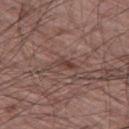workup = catalogued during a skin exam; not biopsied | acquisition = ~15 mm tile from a whole-body skin photo | size = ~3 mm (longest diameter) | illumination = white-light | patient = male, aged around 60 | body site = the left thigh.